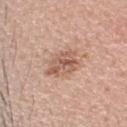{
  "biopsy_status": "not biopsied; imaged during a skin examination",
  "site": "head or neck",
  "lesion_size": {
    "long_diameter_mm_approx": 4.0
  },
  "patient": {
    "sex": "male",
    "age_approx": 35
  },
  "image": {
    "source": "total-body photography crop",
    "field_of_view_mm": 15
  },
  "automated_metrics": {
    "eccentricity": 0.5,
    "shape_asymmetry": 0.25,
    "nevus_likeness_0_100": 5,
    "lesion_detection_confidence_0_100": 100
  }
}